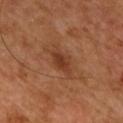Assessment:
The lesion was tiled from a total-body skin photograph and was not biopsied.
Acquisition and patient details:
The total-body-photography lesion software estimated a lesion color around L≈38 a*≈24 b*≈32 in CIELAB, about 9 CIELAB-L* units darker than the surrounding skin, and a normalized lesion–skin contrast near 7.5. A roughly 15 mm field-of-view crop from a total-body skin photograph. A male patient, in their 50s. On the chest. The lesion's longest dimension is about 3.5 mm. Captured under cross-polarized illumination.